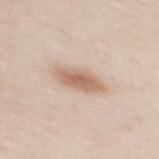workup = catalogued during a skin exam; not biopsied | site = the mid back | acquisition = 15 mm crop, total-body photography | lighting = white-light | subject = female, in their 30s | lesion diameter = ≈5 mm | automated lesion analysis = a lesion area of about 8.5 mm², a shape eccentricity near 0.9, and two-axis asymmetry of about 0.15; a lesion color around L≈64 a*≈17 b*≈29 in CIELAB, a lesion–skin lightness drop of about 12, and a normalized border contrast of about 7.5.